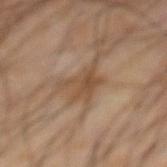Q: Was a biopsy performed?
A: no biopsy performed (imaged during a skin exam)
Q: Lesion size?
A: about 4 mm
Q: What is the anatomic site?
A: the abdomen
Q: Automated lesion metrics?
A: a lesion area of about 7.5 mm², an eccentricity of roughly 0.6, and a shape-asymmetry score of about 0.5 (0 = symmetric); a lesion color around L≈45 a*≈15 b*≈30 in CIELAB and a normalized border contrast of about 6.5; a peripheral color-asymmetry measure near 1
Q: Illumination type?
A: cross-polarized illumination
Q: Who is the patient?
A: male, aged 63–67
Q: What kind of image is this?
A: total-body-photography crop, ~15 mm field of view